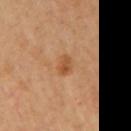Q: Is there a histopathology result?
A: imaged on a skin check; not biopsied
Q: What is the lesion's diameter?
A: about 2.5 mm
Q: Where on the body is the lesion?
A: the left arm
Q: How was this image acquired?
A: ~15 mm tile from a whole-body skin photo
Q: Patient demographics?
A: female, aged 58 to 62
Q: What did automated image analysis measure?
A: a lesion area of about 4 mm², a shape eccentricity near 0.7, and two-axis asymmetry of about 0.15; a nevus-likeness score of about 20/100 and a detector confidence of about 100 out of 100 that the crop contains a lesion
Q: Illumination type?
A: cross-polarized illumination